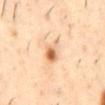automated metrics = a lesion area of about 5.5 mm², a shape eccentricity near 0.8, and two-axis asymmetry of about 0.35; an average lesion color of about L≈67 a*≈21 b*≈38 (CIELAB); border irregularity of about 4 on a 0–10 scale, a color-variation rating of about 7/10, and a peripheral color-asymmetry measure near 2
tile lighting = cross-polarized illumination
imaging modality = 15 mm crop, total-body photography
location = the mid back
patient = male, aged approximately 55